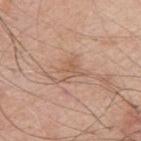Q: Was this lesion biopsied?
A: no biopsy performed (imaged during a skin exam)
Q: What is the imaging modality?
A: ~15 mm tile from a whole-body skin photo
Q: What is the anatomic site?
A: the right upper arm
Q: Who is the patient?
A: male, aged 58 to 62